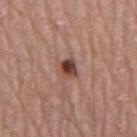location — the mid back
image-analysis metrics — an area of roughly 4 mm², a shape eccentricity near 0.55, and two-axis asymmetry of about 0.3; roughly 15 lightness units darker than nearby skin and a normalized border contrast of about 11; a border-irregularity index near 2.5/10, a color-variation rating of about 7.5/10, and peripheral color asymmetry of about 2.5; a detector confidence of about 100 out of 100 that the crop contains a lesion
acquisition — ~15 mm tile from a whole-body skin photo
lesion diameter — about 2.5 mm
subject — male, approximately 80 years of age